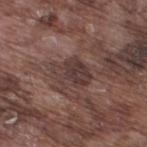Captured during whole-body skin photography for melanoma surveillance; the lesion was not biopsied.
The total-body-photography lesion software estimated a lesion color around L≈36 a*≈17 b*≈19 in CIELAB and a normalized border contrast of about 8. The software also gave a border-irregularity index near 4/10, internal color variation of about 3.5 on a 0–10 scale, and a peripheral color-asymmetry measure near 1.
On the right thigh.
A 15 mm crop from a total-body photograph taken for skin-cancer surveillance.
About 4 mm across.
Imaged with white-light lighting.
A male subject, aged around 75.